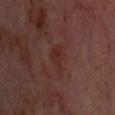This lesion was catalogued during total-body skin photography and was not selected for biopsy. An algorithmic analysis of the crop reported a lesion area of about 5.5 mm², a shape eccentricity near 0.75, and two-axis asymmetry of about 0.5. The software also gave an average lesion color of about L≈27 a*≈21 b*≈22 (CIELAB) and a lesion–skin lightness drop of about 4. The analysis additionally found a classifier nevus-likeness of about 0/100 and a detector confidence of about 100 out of 100 that the crop contains a lesion. Located on the head or neck. About 3.5 mm across. The patient is in their mid- to late 60s. Cropped from a whole-body photographic skin survey; the tile spans about 15 mm. This is a cross-polarized tile.